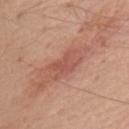Captured during whole-body skin photography for melanoma surveillance; the lesion was not biopsied. The subject is a male roughly 80 years of age. Approximately 4 mm at its widest. On the upper back. Cropped from a total-body skin-imaging series; the visible field is about 15 mm. The tile uses white-light illumination.